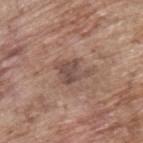notes: imaged on a skin check; not biopsied | size: about 4 mm | imaging modality: total-body-photography crop, ~15 mm field of view | tile lighting: white-light | TBP lesion metrics: an area of roughly 7 mm² and a symmetry-axis asymmetry near 0.45; a border-irregularity index near 5.5/10 and peripheral color asymmetry of about 1; a nevus-likeness score of about 5/100 and a lesion-detection confidence of about 100/100 | site: the upper back | patient: male, in their 70s.